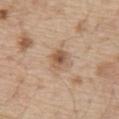Imaged during a routine full-body skin examination; the lesion was not biopsied and no histopathology is available. A region of skin cropped from a whole-body photographic capture, roughly 15 mm wide. A male patient, aged around 70. The lesion's longest dimension is about 2.5 mm. Located on the abdomen.